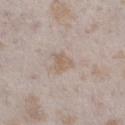| feature | finding |
|---|---|
| biopsy status | no biopsy performed (imaged during a skin exam) |
| image source | ~15 mm crop, total-body skin-cancer survey |
| location | the left lower leg |
| patient | female, aged around 25 |